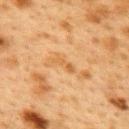Clinical impression: Part of a total-body skin-imaging series; this lesion was reviewed on a skin check and was not flagged for biopsy. Context: A female patient aged approximately 40. The lesion is located on the upper back. This image is a 15 mm lesion crop taken from a total-body photograph.